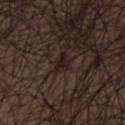Cropped from a total-body skin-imaging series; the visible field is about 15 mm. The lesion is on the abdomen. A male subject, in their mid-70s. About 3 mm across. The tile uses white-light illumination.